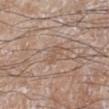follow-up: imaged on a skin check; not biopsied.